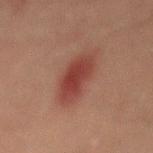Part of a total-body skin-imaging series; this lesion was reviewed on a skin check and was not flagged for biopsy.
A male subject, in their mid-40s.
The lesion's longest dimension is about 6.5 mm.
A 15 mm crop from a total-body photograph taken for skin-cancer surveillance.
The total-body-photography lesion software estimated a footprint of about 14 mm². The software also gave a lesion color around L≈34 a*≈23 b*≈23 in CIELAB and a normalized border contrast of about 8. It also reported a nevus-likeness score of about 100/100 and a lesion-detection confidence of about 100/100.
This is a cross-polarized tile.
Located on the mid back.